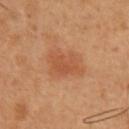Captured during whole-body skin photography for melanoma surveillance; the lesion was not biopsied. An algorithmic analysis of the crop reported a lesion area of about 12 mm², a shape eccentricity near 0.7, and a symmetry-axis asymmetry near 0.25. It also reported an average lesion color of about L≈52 a*≈25 b*≈36 (CIELAB), a lesion–skin lightness drop of about 8, and a normalized border contrast of about 5.5. The software also gave a border-irregularity index near 3/10, internal color variation of about 2.5 on a 0–10 scale, and a peripheral color-asymmetry measure near 0.5. The lesion is located on the chest. A male subject, approximately 50 years of age. The recorded lesion diameter is about 5 mm. A 15 mm close-up tile from a total-body photography series done for melanoma screening. This is a cross-polarized tile.